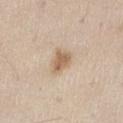Assessment:
Part of a total-body skin-imaging series; this lesion was reviewed on a skin check and was not flagged for biopsy.
Background:
Automated tile analysis of the lesion measured a mean CIELAB color near L≈62 a*≈16 b*≈32 and a lesion-to-skin contrast of about 7.5 (normalized; higher = more distinct). From the right thigh. The subject is a female aged 58 to 62. A roughly 15 mm field-of-view crop from a total-body skin photograph. Approximately 3 mm at its widest. This is a white-light tile.